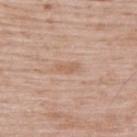{
  "biopsy_status": "not biopsied; imaged during a skin examination",
  "lesion_size": {
    "long_diameter_mm_approx": 2.5
  },
  "patient": {
    "sex": "male",
    "age_approx": 50
  },
  "site": "upper back",
  "image": {
    "source": "total-body photography crop",
    "field_of_view_mm": 15
  },
  "lighting": "white-light"
}